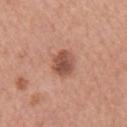biopsy_status: not biopsied; imaged during a skin examination
patient:
  sex: female
  age_approx: 60
lesion_size:
  long_diameter_mm_approx: 3.5
image:
  source: total-body photography crop
  field_of_view_mm: 15
automated_metrics:
  cielab_L: 52
  cielab_a: 24
  cielab_b: 29
  vs_skin_darker_L: 13.0
  vs_skin_contrast_norm: 8.5
  nevus_likeness_0_100: 70
  lesion_detection_confidence_0_100: 100
lighting: white-light
site: right upper arm